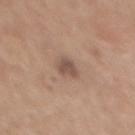Q: Was a biopsy performed?
A: no biopsy performed (imaged during a skin exam)
Q: Lesion location?
A: the back
Q: What kind of image is this?
A: 15 mm crop, total-body photography
Q: Who is the patient?
A: female, aged 58–62